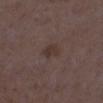| feature | finding |
|---|---|
| follow-up | catalogued during a skin exam; not biopsied |
| acquisition | total-body-photography crop, ~15 mm field of view |
| subject | female, about 35 years old |
| illumination | white-light illumination |
| site | the left lower leg |